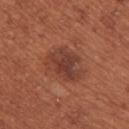Clinical impression: This lesion was catalogued during total-body skin photography and was not selected for biopsy. Clinical summary: The tile uses white-light illumination. Approximately 5.5 mm at its widest. The lesion is located on the right upper arm. A female subject aged 63–67. Cropped from a whole-body photographic skin survey; the tile spans about 15 mm.